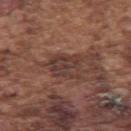follow-up — total-body-photography surveillance lesion; no biopsy | subject — male, aged 73 to 77 | anatomic site — the left upper arm | illumination — white-light | image — 15 mm crop, total-body photography.